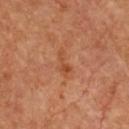The lesion was photographed on a routine skin check and not biopsied; there is no pathology result. This is a cross-polarized tile. An algorithmic analysis of the crop reported a mean CIELAB color near L≈48 a*≈26 b*≈37, a lesion–skin lightness drop of about 6, and a normalized border contrast of about 5.5. It also reported an automated nevus-likeness rating near 0 out of 100. A 15 mm close-up tile from a total-body photography series done for melanoma screening. Measured at roughly 3.5 mm in maximum diameter. From the chest. A female patient approximately 65 years of age.